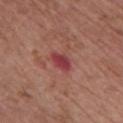notes = no biopsy performed (imaged during a skin exam); lighting = white-light; patient = female, aged 73 to 77; automated lesion analysis = an automated nevus-likeness rating near 0 out of 100 and a detector confidence of about 100 out of 100 that the crop contains a lesion; image source = ~15 mm tile from a whole-body skin photo; anatomic site = the chest; lesion size = ~3.5 mm (longest diameter).